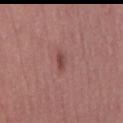This lesion was catalogued during total-body skin photography and was not selected for biopsy.
A female subject in their 50s.
A lesion tile, about 15 mm wide, cut from a 3D total-body photograph.
On the right thigh.
About 3 mm across.
Automated tile analysis of the lesion measured a lesion color around L≈46 a*≈24 b*≈23 in CIELAB, a lesion–skin lightness drop of about 9, and a lesion-to-skin contrast of about 7 (normalized; higher = more distinct).
The tile uses white-light illumination.